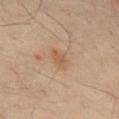The lesion was photographed on a routine skin check and not biopsied; there is no pathology result. Imaged with cross-polarized lighting. On the mid back. Longest diameter approximately 3 mm. The subject is a male aged 63–67. The total-body-photography lesion software estimated a lesion area of about 4.5 mm². It also reported an average lesion color of about L≈53 a*≈16 b*≈32 (CIELAB) and a normalized lesion–skin contrast near 6. It also reported a border-irregularity index near 2.5/10 and internal color variation of about 2.5 on a 0–10 scale. The software also gave a classifier nevus-likeness of about 0/100 and lesion-presence confidence of about 100/100. Cropped from a total-body skin-imaging series; the visible field is about 15 mm.